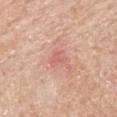| key | value |
|---|---|
| follow-up | total-body-photography surveillance lesion; no biopsy |
| imaging modality | ~15 mm crop, total-body skin-cancer survey |
| body site | the mid back |
| size | ~2.5 mm (longest diameter) |
| patient | male, aged 58 to 62 |
| illumination | white-light illumination |